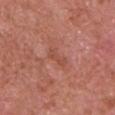Findings:
– notes: imaged on a skin check; not biopsied
– patient: male, aged 63–67
– image source: total-body-photography crop, ~15 mm field of view
– site: the left upper arm
– lighting: white-light illumination